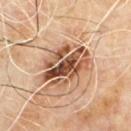Recorded during total-body skin imaging; not selected for excision or biopsy. An algorithmic analysis of the crop reported a lesion area of about 19 mm², an outline eccentricity of about 0.75 (0 = round, 1 = elongated), and a shape-asymmetry score of about 0.15 (0 = symmetric). The software also gave a lesion color around L≈50 a*≈21 b*≈31 in CIELAB and about 16 CIELAB-L* units darker than the surrounding skin. The analysis additionally found border irregularity of about 3 on a 0–10 scale and a within-lesion color-variation index near 10/10. Imaged with cross-polarized lighting. This image is a 15 mm lesion crop taken from a total-body photograph. The lesion is on the back. Approximately 6.5 mm at its widest. A male subject, approximately 60 years of age.